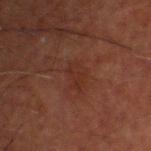Q: Was a biopsy performed?
A: no biopsy performed (imaged during a skin exam)
Q: What lighting was used for the tile?
A: cross-polarized
Q: Lesion size?
A: ~3 mm (longest diameter)
Q: How was this image acquired?
A: 15 mm crop, total-body photography
Q: Lesion location?
A: the head or neck
Q: Patient demographics?
A: male, about 60 years old